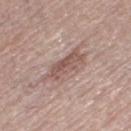Findings:
• biopsy status — total-body-photography surveillance lesion; no biopsy
• size — about 5 mm
• image — total-body-photography crop, ~15 mm field of view
• illumination — white-light illumination
• subject — female, aged 63–67
• location — the right thigh